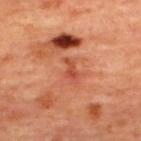Assessment: Captured during whole-body skin photography for melanoma surveillance; the lesion was not biopsied. Context: A female subject aged around 45. This is a cross-polarized tile. The lesion is located on the upper back. The recorded lesion diameter is about 2.5 mm. Cropped from a total-body skin-imaging series; the visible field is about 15 mm.